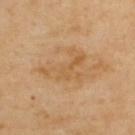image-analysis metrics: a nevus-likeness score of about 0/100 and a detector confidence of about 100 out of 100 that the crop contains a lesion | acquisition: total-body-photography crop, ~15 mm field of view | patient: male, aged approximately 55 | tile lighting: cross-polarized | diameter: ≈5 mm | location: the upper back.